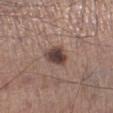Clinical summary: A male patient, aged 53 to 57. On the leg. A 15 mm close-up extracted from a 3D total-body photography capture.